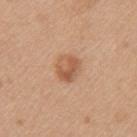This lesion was catalogued during total-body skin photography and was not selected for biopsy. The subject is a female roughly 40 years of age. This is a white-light tile. The lesion-visualizer software estimated a footprint of about 7 mm², a shape eccentricity near 0.5, and a symmetry-axis asymmetry near 0.1. The software also gave a lesion color around L≈57 a*≈22 b*≈34 in CIELAB and a lesion–skin lightness drop of about 10. And it measured internal color variation of about 5.5 on a 0–10 scale and a peripheral color-asymmetry measure near 2. A 15 mm crop from a total-body photograph taken for skin-cancer surveillance. The lesion is located on the left upper arm.